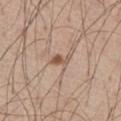notes: catalogued during a skin exam; not biopsied | site: the right thigh | tile lighting: white-light | imaging modality: total-body-photography crop, ~15 mm field of view | size: ≈2.5 mm | patient: male, aged 58–62.